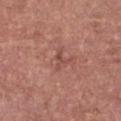Impression: Imaged during a routine full-body skin examination; the lesion was not biopsied and no histopathology is available. Clinical summary: Automated tile analysis of the lesion measured a mean CIELAB color near L≈49 a*≈25 b*≈26, roughly 7 lightness units darker than nearby skin, and a normalized border contrast of about 5.5. A female patient about 45 years old. The lesion is on the chest. Captured under white-light illumination. A 15 mm close-up tile from a total-body photography series done for melanoma screening. Measured at roughly 2.5 mm in maximum diameter.